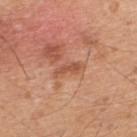Acquisition and patient details: The patient is a male approximately 45 years of age. The lesion-visualizer software estimated an average lesion color of about L≈53 a*≈25 b*≈34 (CIELAB), roughly 9 lightness units darker than nearby skin, and a normalized lesion–skin contrast near 6.5. Measured at roughly 3 mm in maximum diameter. On the upper back. Imaged with white-light lighting. Cropped from a total-body skin-imaging series; the visible field is about 15 mm.